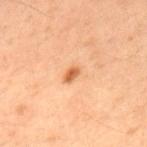  biopsy_status: not biopsied; imaged during a skin examination
  image:
    source: total-body photography crop
    field_of_view_mm: 15
  patient:
    sex: male
    age_approx: 60
  site: back